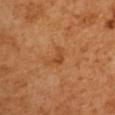workup: no biopsy performed (imaged during a skin exam); patient: male, approximately 50 years of age; imaging modality: ~15 mm tile from a whole-body skin photo; tile lighting: cross-polarized; body site: the right upper arm; diameter: ~2.5 mm (longest diameter).